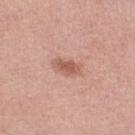Captured during whole-body skin photography for melanoma surveillance; the lesion was not biopsied.
A close-up tile cropped from a whole-body skin photograph, about 15 mm across.
The tile uses white-light illumination.
Automated tile analysis of the lesion measured an average lesion color of about L≈58 a*≈23 b*≈28 (CIELAB), about 10 CIELAB-L* units darker than the surrounding skin, and a normalized border contrast of about 7. It also reported border irregularity of about 2.5 on a 0–10 scale and internal color variation of about 2 on a 0–10 scale. The software also gave a classifier nevus-likeness of about 85/100 and a detector confidence of about 100 out of 100 that the crop contains a lesion.
A male patient aged 48 to 52.
About 3.5 mm across.
From the leg.